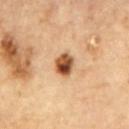Q: Was a biopsy performed?
A: total-body-photography surveillance lesion; no biopsy
Q: What lighting was used for the tile?
A: cross-polarized illumination
Q: What is the lesion's diameter?
A: ~3.5 mm (longest diameter)
Q: Patient demographics?
A: male, aged approximately 85 or older
Q: What is the anatomic site?
A: the upper back
Q: What is the imaging modality?
A: ~15 mm crop, total-body skin-cancer survey
Q: What did automated image analysis measure?
A: an outline eccentricity of about 0.35 (0 = round, 1 = elongated) and a symmetry-axis asymmetry near 0.15; a mean CIELAB color near L≈56 a*≈20 b*≈36, a lesion–skin lightness drop of about 15, and a normalized border contrast of about 10; a border-irregularity rating of about 1.5/10, a within-lesion color-variation index near 10/10, and peripheral color asymmetry of about 3.5